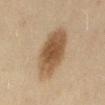On the back. This image is a 15 mm lesion crop taken from a total-body photograph. The patient is a female in their 60s.